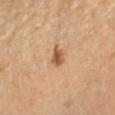| feature | finding |
|---|---|
| workup | no biopsy performed (imaged during a skin exam) |
| acquisition | ~15 mm crop, total-body skin-cancer survey |
| site | the left forearm |
| patient | female, in their 60s |